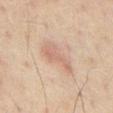acquisition: ~15 mm crop, total-body skin-cancer survey
lighting: cross-polarized illumination
location: the abdomen
patient: male, about 60 years old